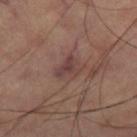  biopsy_status: not biopsied; imaged during a skin examination
  site: leg
  image:
    source: total-body photography crop
    field_of_view_mm: 15
  lesion_size:
    long_diameter_mm_approx: 3.0